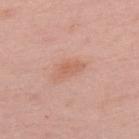Q: Was this lesion biopsied?
A: catalogued during a skin exam; not biopsied
Q: What is the lesion's diameter?
A: ≈3 mm
Q: What are the patient's age and sex?
A: female, aged 48–52
Q: Illumination type?
A: white-light
Q: What kind of image is this?
A: 15 mm crop, total-body photography
Q: What is the anatomic site?
A: the upper back